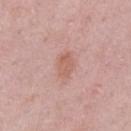The lesion was tiled from a total-body skin photograph and was not biopsied.
The lesion's longest dimension is about 3 mm.
Automated image analysis of the tile measured an eccentricity of roughly 0.8. It also reported an average lesion color of about L≈60 a*≈23 b*≈27 (CIELAB) and a lesion–skin lightness drop of about 8. And it measured a border-irregularity rating of about 2.5/10, internal color variation of about 2 on a 0–10 scale, and a peripheral color-asymmetry measure near 0.5. The software also gave a nevus-likeness score of about 15/100 and a detector confidence of about 100 out of 100 that the crop contains a lesion.
A 15 mm crop from a total-body photograph taken for skin-cancer surveillance.
The lesion is on the left upper arm.
The patient is a female approximately 30 years of age.
This is a white-light tile.